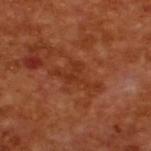Imaged with cross-polarized lighting. A lesion tile, about 15 mm wide, cut from a 3D total-body photograph. Measured at roughly 5.5 mm in maximum diameter. A male subject about 65 years old.